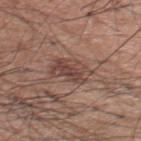A 15 mm crop from a total-body photograph taken for skin-cancer surveillance. A male subject aged 58–62. The total-body-photography lesion software estimated a lesion area of about 9.5 mm², an eccentricity of roughly 0.8, and a symmetry-axis asymmetry near 0.25. The software also gave an average lesion color of about L≈44 a*≈19 b*≈23 (CIELAB), roughly 9 lightness units darker than nearby skin, and a lesion-to-skin contrast of about 7.5 (normalized; higher = more distinct). And it measured internal color variation of about 4.5 on a 0–10 scale and peripheral color asymmetry of about 1.5. The analysis additionally found a classifier nevus-likeness of about 5/100 and lesion-presence confidence of about 100/100. The tile uses white-light illumination. On the upper back.